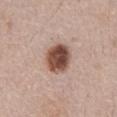Q: Was this lesion biopsied?
A: catalogued during a skin exam; not biopsied
Q: What is the imaging modality?
A: 15 mm crop, total-body photography
Q: Where on the body is the lesion?
A: the abdomen
Q: Who is the patient?
A: male, approximately 65 years of age